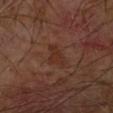No biopsy was performed on this lesion — it was imaged during a full skin examination and was not determined to be concerning.
The lesion is on the left forearm.
The total-body-photography lesion software estimated a footprint of about 5 mm², an outline eccentricity of about 0.8 (0 = round, 1 = elongated), and a shape-asymmetry score of about 0.4 (0 = symmetric). And it measured a lesion color around L≈29 a*≈20 b*≈25 in CIELAB, about 5 CIELAB-L* units darker than the surrounding skin, and a normalized border contrast of about 5.5. The analysis additionally found border irregularity of about 4 on a 0–10 scale.
Imaged with cross-polarized lighting.
Longest diameter approximately 3.5 mm.
A male patient, aged around 70.
A lesion tile, about 15 mm wide, cut from a 3D total-body photograph.